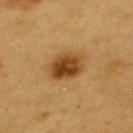| field | value |
|---|---|
| follow-up | total-body-photography surveillance lesion; no biopsy |
| acquisition | 15 mm crop, total-body photography |
| subject | male, aged 58–62 |
| location | the upper back |